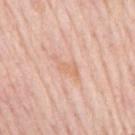The lesion was tiled from a total-body skin photograph and was not biopsied.
Cropped from a whole-body photographic skin survey; the tile spans about 15 mm.
The recorded lesion diameter is about 3.5 mm.
The lesion is located on the mid back.
The subject is a male aged 78–82.